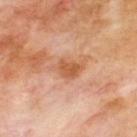The lesion was photographed on a routine skin check and not biopsied; there is no pathology result.
An algorithmic analysis of the crop reported an eccentricity of roughly 0.75. The analysis additionally found an average lesion color of about L≈57 a*≈24 b*≈36 (CIELAB), about 9 CIELAB-L* units darker than the surrounding skin, and a normalized lesion–skin contrast near 7. The software also gave a lesion-detection confidence of about 100/100.
A male patient aged 68 to 72.
From the upper back.
This is a cross-polarized tile.
Cropped from a total-body skin-imaging series; the visible field is about 15 mm.